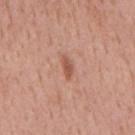Clinical impression:
Captured during whole-body skin photography for melanoma surveillance; the lesion was not biopsied.
Acquisition and patient details:
Located on the mid back. The subject is a male about 55 years old. The recorded lesion diameter is about 3 mm. Captured under white-light illumination. A region of skin cropped from a whole-body photographic capture, roughly 15 mm wide.